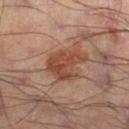notes = total-body-photography surveillance lesion; no biopsy
lesion diameter = ~5 mm (longest diameter)
subject = male, aged 53 to 57
image-analysis metrics = a classifier nevus-likeness of about 25/100 and lesion-presence confidence of about 100/100
body site = the right lower leg
imaging modality = 15 mm crop, total-body photography